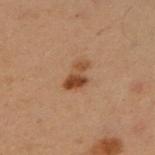Captured during whole-body skin photography for melanoma surveillance; the lesion was not biopsied. A female patient, about 50 years old. Approximately 3.5 mm at its widest. Imaged with cross-polarized lighting. This image is a 15 mm lesion crop taken from a total-body photograph. The lesion is on the arm. The lesion-visualizer software estimated a symmetry-axis asymmetry near 0.45. It also reported border irregularity of about 5 on a 0–10 scale, a within-lesion color-variation index near 3/10, and peripheral color asymmetry of about 0.5.